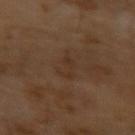Impression: Captured during whole-body skin photography for melanoma surveillance; the lesion was not biopsied. Acquisition and patient details: A male patient in their mid- to late 60s. A lesion tile, about 15 mm wide, cut from a 3D total-body photograph.